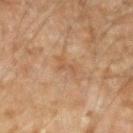Findings:
• follow-up — no biopsy performed (imaged during a skin exam)
• size — about 3 mm
• image — ~15 mm tile from a whole-body skin photo
• body site — the left forearm
• subject — male, aged around 45
• tile lighting — cross-polarized
• image-analysis metrics — a shape eccentricity near 0.9 and a shape-asymmetry score of about 0.55 (0 = symmetric); a nevus-likeness score of about 0/100 and a detector confidence of about 100 out of 100 that the crop contains a lesion